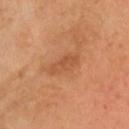Case summary:
* notes: imaged on a skin check; not biopsied
* patient: male, in their mid-40s
* anatomic site: the head or neck
* imaging modality: ~15 mm crop, total-body skin-cancer survey